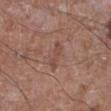Imaged during a routine full-body skin examination; the lesion was not biopsied and no histopathology is available.
The subject is a male aged approximately 65.
The lesion's longest dimension is about 3.5 mm.
A 15 mm close-up extracted from a 3D total-body photography capture.
The lesion is on the leg.
Automated tile analysis of the lesion measured an average lesion color of about L≈47 a*≈21 b*≈25 (CIELAB) and a lesion-to-skin contrast of about 5 (normalized; higher = more distinct). And it measured border irregularity of about 5 on a 0–10 scale and a peripheral color-asymmetry measure near 0.